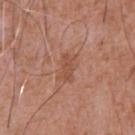Assessment:
Captured during whole-body skin photography for melanoma surveillance; the lesion was not biopsied.
Image and clinical context:
A region of skin cropped from a whole-body photographic capture, roughly 15 mm wide. A male subject about 75 years old. From the chest. This is a white-light tile.